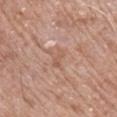Clinical impression: Part of a total-body skin-imaging series; this lesion was reviewed on a skin check and was not flagged for biopsy. Acquisition and patient details: A male patient aged around 75. The lesion is located on the right lower leg. A lesion tile, about 15 mm wide, cut from a 3D total-body photograph. This is a white-light tile. About 2.5 mm across.